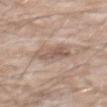  biopsy_status: not biopsied; imaged during a skin examination
  lighting: white-light
  automated_metrics:
    nevus_likeness_0_100: 0
  image:
    source: total-body photography crop
    field_of_view_mm: 15
  patient:
    sex: male
    age_approx: 60
  site: chest
  lesion_size:
    long_diameter_mm_approx: 3.5